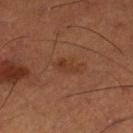Notes:
* patient · male, approximately 50 years of age
* diameter · ≈3.5 mm
* tile lighting · cross-polarized
* site · the left lower leg
* image · 15 mm crop, total-body photography